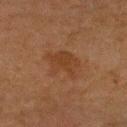Q: Is there a histopathology result?
A: total-body-photography surveillance lesion; no biopsy
Q: What are the patient's age and sex?
A: male, roughly 60 years of age
Q: Automated lesion metrics?
A: an outline eccentricity of about 0.55 (0 = round, 1 = elongated); an average lesion color of about L≈33 a*≈18 b*≈28 (CIELAB), about 5 CIELAB-L* units darker than the surrounding skin, and a normalized lesion–skin contrast near 5.5; a border-irregularity index near 4/10, a color-variation rating of about 2/10, and a peripheral color-asymmetry measure near 0.5; a classifier nevus-likeness of about 15/100 and a detector confidence of about 100 out of 100 that the crop contains a lesion
Q: Lesion size?
A: about 4 mm
Q: How was this image acquired?
A: total-body-photography crop, ~15 mm field of view
Q: What is the anatomic site?
A: the head or neck